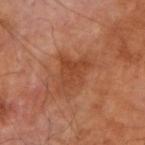Q: Was this lesion biopsied?
A: catalogued during a skin exam; not biopsied
Q: Where on the body is the lesion?
A: the arm
Q: What kind of image is this?
A: ~15 mm crop, total-body skin-cancer survey
Q: What are the patient's age and sex?
A: male, aged around 70
Q: What lighting was used for the tile?
A: cross-polarized illumination